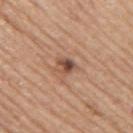follow-up: no biopsy performed (imaged during a skin exam) | image source: total-body-photography crop, ~15 mm field of view | patient: male, aged 73–77 | size: ≈2.5 mm | lighting: white-light illumination | body site: the right upper arm.